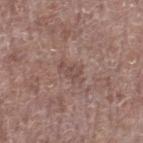{"biopsy_status": "not biopsied; imaged during a skin examination", "automated_metrics": {"eccentricity": 0.85, "shape_asymmetry": 0.4, "vs_skin_darker_L": 7.0, "vs_skin_contrast_norm": 5.0}, "lesion_size": {"long_diameter_mm_approx": 3.0}, "site": "right lower leg", "patient": {"sex": "male", "age_approx": 70}, "image": {"source": "total-body photography crop", "field_of_view_mm": 15}, "lighting": "white-light"}